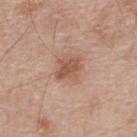- notes: imaged on a skin check; not biopsied
- size: ≈3 mm
- patient: male, in their mid-60s
- image: ~15 mm crop, total-body skin-cancer survey
- location: the upper back
- TBP lesion metrics: an area of roughly 5 mm², an eccentricity of roughly 0.7, and a symmetry-axis asymmetry near 0.2; a mean CIELAB color near L≈54 a*≈21 b*≈30, about 10 CIELAB-L* units darker than the surrounding skin, and a normalized border contrast of about 7.5; a border-irregularity rating of about 2/10, internal color variation of about 2 on a 0–10 scale, and radial color variation of about 1
- illumination: white-light illumination